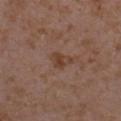The lesion was tiled from a total-body skin photograph and was not biopsied.
A 15 mm close-up extracted from a 3D total-body photography capture.
The lesion is located on the arm.
Longest diameter approximately 3 mm.
The patient is a female aged 33 to 37.
Captured under white-light illumination.